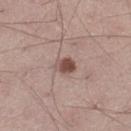Notes:
* notes: catalogued during a skin exam; not biopsied
* automated metrics: a footprint of about 4 mm²; an average lesion color of about L≈48 a*≈19 b*≈23 (CIELAB), about 14 CIELAB-L* units darker than the surrounding skin, and a normalized border contrast of about 9.5; a nevus-likeness score of about 95/100 and a lesion-detection confidence of about 100/100
* illumination: white-light
* diameter: about 2.5 mm
* image source: ~15 mm crop, total-body skin-cancer survey
* subject: male, about 45 years old
* body site: the left thigh